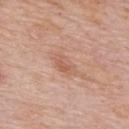workup: total-body-photography surveillance lesion; no biopsy
location: the back
size: ≈3.5 mm
illumination: white-light
patient: male, in their mid- to late 70s
imaging modality: 15 mm crop, total-body photography
image-analysis metrics: a footprint of about 5 mm² and a shape eccentricity near 0.85; a lesion color around L≈59 a*≈22 b*≈30 in CIELAB, about 7 CIELAB-L* units darker than the surrounding skin, and a normalized lesion–skin contrast near 5; border irregularity of about 2.5 on a 0–10 scale, a within-lesion color-variation index near 2.5/10, and peripheral color asymmetry of about 0.5